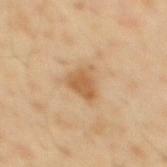{
  "biopsy_status": "not biopsied; imaged during a skin examination",
  "image": {
    "source": "total-body photography crop",
    "field_of_view_mm": 15
  },
  "automated_metrics": {
    "cielab_L": 59,
    "cielab_a": 20,
    "cielab_b": 39,
    "vs_skin_darker_L": 10.0,
    "vs_skin_contrast_norm": 7.5,
    "nevus_likeness_0_100": 40,
    "lesion_detection_confidence_0_100": 100
  },
  "lesion_size": {
    "long_diameter_mm_approx": 3.5
  },
  "site": "mid back",
  "patient": {
    "sex": "male",
    "age_approx": 40
  }
}